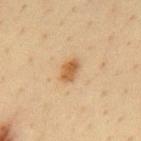Impression: No biopsy was performed on this lesion — it was imaged during a full skin examination and was not determined to be concerning. Context: Automated tile analysis of the lesion measured a lesion area of about 4.5 mm², an eccentricity of roughly 0.75, and a shape-asymmetry score of about 0.15 (0 = symmetric). The software also gave an average lesion color of about L≈54 a*≈19 b*≈37 (CIELAB) and a lesion-to-skin contrast of about 8.5 (normalized; higher = more distinct). And it measured border irregularity of about 1.5 on a 0–10 scale and peripheral color asymmetry of about 1. The lesion is on the mid back. The lesion's longest dimension is about 3 mm. A male subject in their mid- to late 30s. A region of skin cropped from a whole-body photographic capture, roughly 15 mm wide. The tile uses cross-polarized illumination.